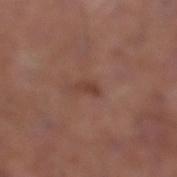biopsy_status: not biopsied; imaged during a skin examination
image:
  source: total-body photography crop
  field_of_view_mm: 15
automated_metrics:
  cielab_L: 40
  cielab_a: 22
  cielab_b: 26
  vs_skin_darker_L: 7.0
  vs_skin_contrast_norm: 6.5
patient:
  sex: male
  age_approx: 65
site: right lower leg
lesion_size:
  long_diameter_mm_approx: 2.5
lighting: white-light